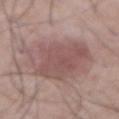{
  "biopsy_status": "not biopsied; imaged during a skin examination",
  "site": "front of the torso",
  "image": {
    "source": "total-body photography crop",
    "field_of_view_mm": 15
  },
  "lighting": "white-light",
  "patient": {
    "sex": "male",
    "age_approx": 70
  },
  "lesion_size": {
    "long_diameter_mm_approx": 7.5
  }
}